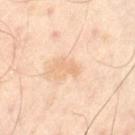follow-up: imaged on a skin check; not biopsied
illumination: cross-polarized
imaging modality: total-body-photography crop, ~15 mm field of view
anatomic site: the lower back
diameter: ~2.5 mm (longest diameter)
subject: male, aged 48–52